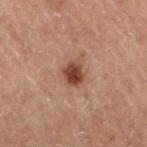{"biopsy_status": "not biopsied; imaged during a skin examination", "image": {"source": "total-body photography crop", "field_of_view_mm": 15}, "lighting": "cross-polarized", "lesion_size": {"long_diameter_mm_approx": 3.0}, "site": "right thigh", "patient": {"sex": "male", "age_approx": 70}}